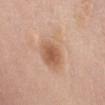Findings:
* subject · female, about 60 years old
* TBP lesion metrics · a shape eccentricity near 0.7 and two-axis asymmetry of about 0.3; a lesion color around L≈62 a*≈19 b*≈32 in CIELAB, about 8 CIELAB-L* units darker than the surrounding skin, and a normalized border contrast of about 5.5; border irregularity of about 3 on a 0–10 scale, a within-lesion color-variation index near 6/10, and a peripheral color-asymmetry measure near 1.5; a nevus-likeness score of about 90/100 and a detector confidence of about 100 out of 100 that the crop contains a lesion
* location · the abdomen
* image source · 15 mm crop, total-body photography
* lesion diameter · about 6 mm